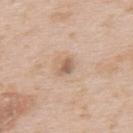No biopsy was performed on this lesion — it was imaged during a full skin examination and was not determined to be concerning.